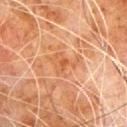Notes:
- workup — catalogued during a skin exam; not biopsied
- patient — male, in their 80s
- location — the chest
- image — ~15 mm tile from a whole-body skin photo